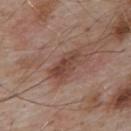| field | value |
|---|---|
| notes | imaged on a skin check; not biopsied |
| diameter | ≈4.5 mm |
| illumination | white-light illumination |
| subject | male, roughly 55 years of age |
| imaging modality | 15 mm crop, total-body photography |
| anatomic site | the upper back |